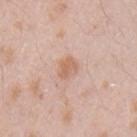Clinical impression:
Imaged during a routine full-body skin examination; the lesion was not biopsied and no histopathology is available.
Background:
The patient is a male aged approximately 25. This is a white-light tile. This image is a 15 mm lesion crop taken from a total-body photograph. From the arm.